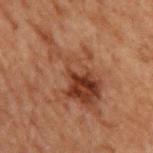Assessment: Imaged during a routine full-body skin examination; the lesion was not biopsied and no histopathology is available. Background: Captured under cross-polarized illumination. Measured at roughly 10 mm in maximum diameter. A 15 mm crop from a total-body photograph taken for skin-cancer surveillance. A male patient aged 68 to 72. On the back. Automated tile analysis of the lesion measured about 8 CIELAB-L* units darker than the surrounding skin and a normalized lesion–skin contrast near 7.5. It also reported border irregularity of about 9 on a 0–10 scale, internal color variation of about 8.5 on a 0–10 scale, and radial color variation of about 3.